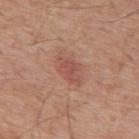Assessment:
Part of a total-body skin-imaging series; this lesion was reviewed on a skin check and was not flagged for biopsy.
Clinical summary:
A 15 mm close-up extracted from a 3D total-body photography capture. A male subject, aged 58–62. Longest diameter approximately 4 mm. Imaged with white-light lighting. The lesion-visualizer software estimated an automated nevus-likeness rating near 20 out of 100 and a lesion-detection confidence of about 100/100. On the back.